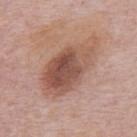image-analysis metrics: a border-irregularity index near 2.5/10 and a color-variation rating of about 7.5/10; a classifier nevus-likeness of about 5/100 and a detector confidence of about 100 out of 100 that the crop contains a lesion | site: the mid back | image source: ~15 mm tile from a whole-body skin photo | patient: male, in their mid-70s | illumination: white-light illumination | lesion diameter: ≈9 mm.